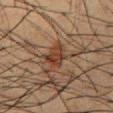This lesion was catalogued during total-body skin photography and was not selected for biopsy. Approximately 3.5 mm at its widest. A male subject about 35 years old. A lesion tile, about 15 mm wide, cut from a 3D total-body photograph. The lesion-visualizer software estimated a lesion area of about 5.5 mm² and a shape eccentricity near 0.7. And it measured a mean CIELAB color near L≈29 a*≈17 b*≈24, a lesion–skin lightness drop of about 8, and a lesion-to-skin contrast of about 8.5 (normalized; higher = more distinct). Captured under cross-polarized illumination. The lesion is on the chest.